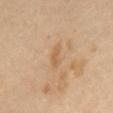Q: Lesion size?
A: about 3 mm
Q: Illumination type?
A: cross-polarized
Q: Who is the patient?
A: female, about 55 years old
Q: What is the imaging modality?
A: ~15 mm crop, total-body skin-cancer survey
Q: What is the anatomic site?
A: the front of the torso
Q: Automated lesion metrics?
A: a lesion area of about 3 mm² and an outline eccentricity of about 0.9 (0 = round, 1 = elongated); about 7 CIELAB-L* units darker than the surrounding skin and a normalized lesion–skin contrast near 5.5